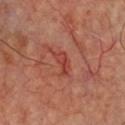Findings:
- automated metrics: an area of roughly 4.5 mm², an outline eccentricity of about 0.85 (0 = round, 1 = elongated), and two-axis asymmetry of about 0.35; a mean CIELAB color near L≈43 a*≈32 b*≈30; internal color variation of about 5 on a 0–10 scale and radial color variation of about 2.5; an automated nevus-likeness rating near 0 out of 100 and lesion-presence confidence of about 95/100
- acquisition: ~15 mm tile from a whole-body skin photo
- lighting: cross-polarized illumination
- anatomic site: the front of the torso
- patient: aged 63–67